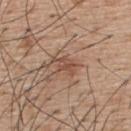notes = total-body-photography surveillance lesion; no biopsy | illumination = white-light illumination | subject = male, in their mid-50s | acquisition = 15 mm crop, total-body photography | location = the upper back.